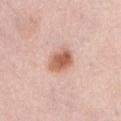follow-up: catalogued during a skin exam; not biopsied.